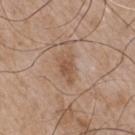No biopsy was performed on this lesion — it was imaged during a full skin examination and was not determined to be concerning. A 15 mm crop from a total-body photograph taken for skin-cancer surveillance. A male patient, in their mid- to late 60s. Automated tile analysis of the lesion measured a shape-asymmetry score of about 0.35 (0 = symmetric). It also reported an automated nevus-likeness rating near 0 out of 100 and a lesion-detection confidence of about 100/100. This is a white-light tile. The lesion is on the chest. About 3.5 mm across.